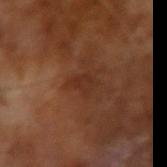Imaged during a routine full-body skin examination; the lesion was not biopsied and no histopathology is available.
Cropped from a total-body skin-imaging series; the visible field is about 15 mm.
A male subject in their mid-60s.
Captured under cross-polarized illumination.